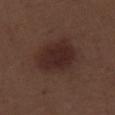A male subject approximately 70 years of age. Approximately 5.5 mm at its widest. The lesion is on the right thigh. A region of skin cropped from a whole-body photographic capture, roughly 15 mm wide. The total-body-photography lesion software estimated a lesion color around L≈26 a*≈17 b*≈19 in CIELAB, about 8 CIELAB-L* units darker than the surrounding skin, and a normalized lesion–skin contrast near 8.5. It also reported border irregularity of about 1.5 on a 0–10 scale, a color-variation rating of about 3/10, and a peripheral color-asymmetry measure near 1. The software also gave a classifier nevus-likeness of about 95/100.